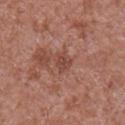| feature | finding |
|---|---|
| follow-up | catalogued during a skin exam; not biopsied |
| site | the right upper arm |
| image source | total-body-photography crop, ~15 mm field of view |
| size | ≈2.5 mm |
| automated lesion analysis | a footprint of about 4.5 mm², an outline eccentricity of about 0.55 (0 = round, 1 = elongated), and a symmetry-axis asymmetry near 0.3; a lesion color around L≈46 a*≈24 b*≈26 in CIELAB, roughly 8 lightness units darker than nearby skin, and a lesion-to-skin contrast of about 6 (normalized; higher = more distinct); a border-irregularity rating of about 2.5/10, a color-variation rating of about 2/10, and radial color variation of about 0.5; a nevus-likeness score of about 0/100 and a detector confidence of about 100 out of 100 that the crop contains a lesion |
| subject | male, approximately 65 years of age |